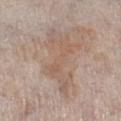subject: male, approximately 60 years of age
anatomic site: the right lower leg
diameter: about 7.5 mm
illumination: white-light illumination
imaging modality: ~15 mm crop, total-body skin-cancer survey